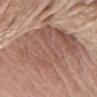The lesion was tiled from a total-body skin photograph and was not biopsied. A female patient aged around 75. A roughly 15 mm field-of-view crop from a total-body skin photograph. On the right upper arm. An algorithmic analysis of the crop reported a lesion area of about 60 mm² and a shape eccentricity near 0.85. Imaged with white-light lighting. Approximately 13 mm at its widest.